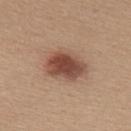| field | value |
|---|---|
| patient | female, aged around 30 |
| imaging modality | 15 mm crop, total-body photography |
| anatomic site | the upper back |